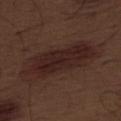Impression:
This lesion was catalogued during total-body skin photography and was not selected for biopsy.
Background:
A region of skin cropped from a whole-body photographic capture, roughly 15 mm wide. The lesion is located on the leg. The lesion-visualizer software estimated a lesion area of about 27 mm², an outline eccentricity of about 0.95 (0 = round, 1 = elongated), and two-axis asymmetry of about 0.2. It also reported a lesion color around L≈23 a*≈18 b*≈19 in CIELAB, about 7 CIELAB-L* units darker than the surrounding skin, and a normalized border contrast of about 9. The software also gave a border-irregularity rating of about 4/10, internal color variation of about 3.5 on a 0–10 scale, and a peripheral color-asymmetry measure near 1. The analysis additionally found a nevus-likeness score of about 50/100 and lesion-presence confidence of about 100/100. Captured under white-light illumination. A male subject about 70 years old. The recorded lesion diameter is about 10.5 mm.